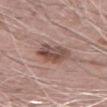Q: Is there a histopathology result?
A: catalogued during a skin exam; not biopsied
Q: What is the anatomic site?
A: the right upper arm
Q: What kind of image is this?
A: ~15 mm tile from a whole-body skin photo
Q: Patient demographics?
A: male, roughly 70 years of age